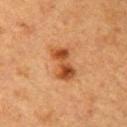Context: An algorithmic analysis of the crop reported roughly 12 lightness units darker than nearby skin. A female patient aged around 70. Captured under cross-polarized illumination. On the right forearm. The recorded lesion diameter is about 4.5 mm. Cropped from a whole-body photographic skin survey; the tile spans about 15 mm.